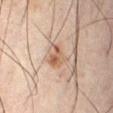notes = catalogued during a skin exam; not biopsied | image-analysis metrics = a lesion area of about 4 mm², an eccentricity of roughly 0.8, and a symmetry-axis asymmetry near 0.45; about 11 CIELAB-L* units darker than the surrounding skin and a normalized border contrast of about 8; a border-irregularity index near 4.5/10, internal color variation of about 5 on a 0–10 scale, and radial color variation of about 2; lesion-presence confidence of about 100/100 | acquisition = ~15 mm tile from a whole-body skin photo | anatomic site = the abdomen | lesion size = about 3 mm | subject = male, approximately 65 years of age | tile lighting = cross-polarized illumination.